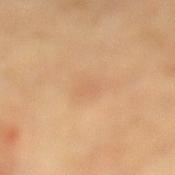The lesion was tiled from a total-body skin photograph and was not biopsied. A close-up tile cropped from a whole-body skin photograph, about 15 mm across. A female patient, roughly 70 years of age. Captured under cross-polarized illumination. Measured at roughly 2.5 mm in maximum diameter. From the left lower leg.